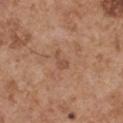This lesion was catalogued during total-body skin photography and was not selected for biopsy.
Imaged with white-light lighting.
Longest diameter approximately 2.5 mm.
A male patient aged around 55.
The lesion-visualizer software estimated an area of roughly 2.5 mm², an outline eccentricity of about 0.85 (0 = round, 1 = elongated), and a symmetry-axis asymmetry near 0.6. It also reported a mean CIELAB color near L≈50 a*≈22 b*≈31, roughly 7 lightness units darker than nearby skin, and a lesion-to-skin contrast of about 5 (normalized; higher = more distinct).
Located on the left upper arm.
A 15 mm crop from a total-body photograph taken for skin-cancer surveillance.